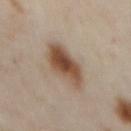notes: imaged on a skin check; not biopsied | imaging modality: ~15 mm tile from a whole-body skin photo | lesion diameter: about 5.5 mm | subject: female, aged approximately 60 | tile lighting: cross-polarized illumination | site: the chest.